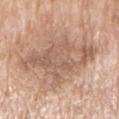Q: Was this lesion biopsied?
A: no biopsy performed (imaged during a skin exam)
Q: Automated lesion metrics?
A: an average lesion color of about L≈59 a*≈19 b*≈28 (CIELAB) and roughly 9 lightness units darker than nearby skin
Q: Where on the body is the lesion?
A: the left upper arm
Q: How was this image acquired?
A: 15 mm crop, total-body photography
Q: Who is the patient?
A: male, aged 68 to 72
Q: Lesion size?
A: ≈8 mm